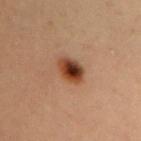notes: no biopsy performed (imaged during a skin exam) | imaging modality: ~15 mm tile from a whole-body skin photo | lighting: cross-polarized | site: the chest | size: ~3.5 mm (longest diameter) | subject: female, aged 38 to 42.